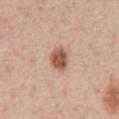Findings:
• follow-up · total-body-photography surveillance lesion; no biopsy
• body site · the front of the torso
• tile lighting · white-light
• lesion size · ~3 mm (longest diameter)
• patient · male, in their mid-50s
• image · ~15 mm crop, total-body skin-cancer survey